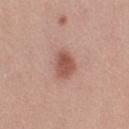- workup: total-body-photography surveillance lesion; no biopsy
- body site: the right thigh
- patient: female, aged around 35
- image: ~15 mm tile from a whole-body skin photo